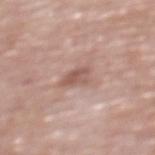Q: Is there a histopathology result?
A: total-body-photography surveillance lesion; no biopsy
Q: What are the patient's age and sex?
A: female, roughly 65 years of age
Q: What is the imaging modality?
A: 15 mm crop, total-body photography
Q: What is the lesion's diameter?
A: ~3 mm (longest diameter)
Q: What is the anatomic site?
A: the chest
Q: How was the tile lit?
A: white-light